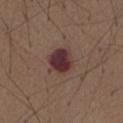On the abdomen. About 3.5 mm across. A male subject aged approximately 75. The tile uses white-light illumination. Cropped from a whole-body photographic skin survey; the tile spans about 15 mm.